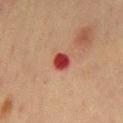Q: Was this lesion biopsied?
A: catalogued during a skin exam; not biopsied
Q: Lesion location?
A: the chest
Q: What is the imaging modality?
A: 15 mm crop, total-body photography
Q: What are the patient's age and sex?
A: male, approximately 70 years of age
Q: Automated lesion metrics?
A: a lesion color around L≈35 a*≈32 b*≈26 in CIELAB, a lesion–skin lightness drop of about 15, and a lesion-to-skin contrast of about 12.5 (normalized; higher = more distinct); a border-irregularity index near 1.5/10, a within-lesion color-variation index near 2/10, and a peripheral color-asymmetry measure near 0.5; a lesion-detection confidence of about 100/100
Q: What is the lesion's diameter?
A: ≈2.5 mm